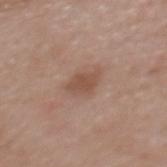The lesion was tiled from a total-body skin photograph and was not biopsied. A close-up tile cropped from a whole-body skin photograph, about 15 mm across. Imaged with white-light lighting. A female subject, in their 60s. Longest diameter approximately 3.5 mm. The lesion is located on the upper back.